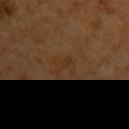Captured during whole-body skin photography for melanoma surveillance; the lesion was not biopsied. Captured under cross-polarized illumination. The lesion-visualizer software estimated a footprint of about 2 mm², an eccentricity of roughly 0.95, and a shape-asymmetry score of about 0.45 (0 = symmetric). The software also gave about 4 CIELAB-L* units darker than the surrounding skin and a lesion-to-skin contrast of about 4.5 (normalized; higher = more distinct). The analysis additionally found a border-irregularity rating of about 5/10, a within-lesion color-variation index near 0/10, and radial color variation of about 0. Longest diameter approximately 2.5 mm. On the arm. Cropped from a total-body skin-imaging series; the visible field is about 15 mm. The subject is a female in their mid- to late 50s.